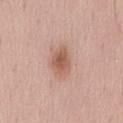Impression:
Captured during whole-body skin photography for melanoma surveillance; the lesion was not biopsied.
Clinical summary:
The lesion is on the lower back. The tile uses white-light illumination. A male subject, in their mid-50s. Automated tile analysis of the lesion measured an automated nevus-likeness rating near 80 out of 100. Longest diameter approximately 3.5 mm. A 15 mm crop from a total-body photograph taken for skin-cancer surveillance.